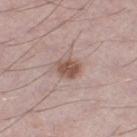workup: total-body-photography surveillance lesion; no biopsy
location: the left thigh
image-analysis metrics: a footprint of about 5 mm², a shape eccentricity near 0.65, and a symmetry-axis asymmetry near 0.2; an average lesion color of about L≈52 a*≈19 b*≈25 (CIELAB) and a lesion–skin lightness drop of about 12; a color-variation rating of about 2.5/10 and a peripheral color-asymmetry measure near 1; lesion-presence confidence of about 100/100
imaging modality: ~15 mm tile from a whole-body skin photo
patient: male, aged 48–52
lesion size: about 3 mm
lighting: white-light